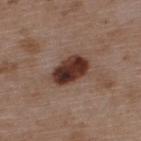Clinical impression: No biopsy was performed on this lesion — it was imaged during a full skin examination and was not determined to be concerning. Clinical summary: The lesion is located on the upper back. Cropped from a whole-body photographic skin survey; the tile spans about 15 mm. Measured at roughly 4.5 mm in maximum diameter. The subject is a male aged 48–52. The lesion-visualizer software estimated a footprint of about 10 mm², an eccentricity of roughly 0.8, and a shape-asymmetry score of about 0.15 (0 = symmetric). And it measured an average lesion color of about L≈33 a*≈20 b*≈24 (CIELAB), roughly 17 lightness units darker than nearby skin, and a normalized border contrast of about 14.5.